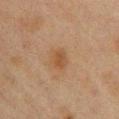Recorded during total-body skin imaging; not selected for excision or biopsy. The subject is a female roughly 40 years of age. Automated tile analysis of the lesion measured an area of roughly 4.5 mm². The analysis additionally found a classifier nevus-likeness of about 80/100. On the chest. Imaged with cross-polarized lighting. The lesion's longest dimension is about 2.5 mm. Cropped from a whole-body photographic skin survey; the tile spans about 15 mm.